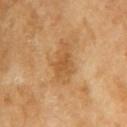biopsy status — no biopsy performed (imaged during a skin exam)
location — the right upper arm
patient — female, approximately 70 years of age
lighting — cross-polarized
imaging modality — 15 mm crop, total-body photography
automated metrics — an eccentricity of roughly 0.8 and two-axis asymmetry of about 0.25; a lesion color around L≈57 a*≈21 b*≈42 in CIELAB, roughly 8 lightness units darker than nearby skin, and a normalized lesion–skin contrast near 6; a color-variation rating of about 3/10 and a peripheral color-asymmetry measure near 0.5; an automated nevus-likeness rating near 0 out of 100 and a lesion-detection confidence of about 100/100
lesion diameter — ≈4.5 mm